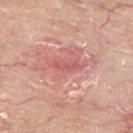Recorded during total-body skin imaging; not selected for excision or biopsy.
The recorded lesion diameter is about 3.5 mm.
On the upper back.
The tile uses white-light illumination.
The subject is a male roughly 65 years of age.
The total-body-photography lesion software estimated an area of roughly 3.5 mm², an outline eccentricity of about 0.95 (0 = round, 1 = elongated), and a shape-asymmetry score of about 0.4 (0 = symmetric). The analysis additionally found a nevus-likeness score of about 0/100.
A 15 mm close-up extracted from a 3D total-body photography capture.